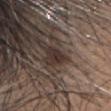Image and clinical context:
The lesion-visualizer software estimated a lesion area of about 3.5 mm², an eccentricity of roughly 0.75, and two-axis asymmetry of about 0.25. It also reported a lesion color around L≈30 a*≈13 b*≈17 in CIELAB, roughly 9 lightness units darker than nearby skin, and a normalized border contrast of about 9.5. And it measured a border-irregularity index near 2.5/10. It also reported a nevus-likeness score of about 20/100. A female subject, aged 38 to 42. Located on the head or neck. Cropped from a whole-body photographic skin survey; the tile spans about 15 mm.